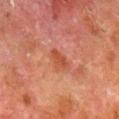This lesion was catalogued during total-body skin photography and was not selected for biopsy. A male subject, aged approximately 80. From the right lower leg. Cropped from a whole-body photographic skin survey; the tile spans about 15 mm.